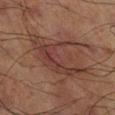Impression:
The lesion was tiled from a total-body skin photograph and was not biopsied.
Acquisition and patient details:
The tile uses cross-polarized illumination. A 15 mm close-up extracted from a 3D total-body photography capture. The patient is a male aged around 70. The lesion-visualizer software estimated an average lesion color of about L≈30 a*≈17 b*≈20 (CIELAB), roughly 6 lightness units darker than nearby skin, and a normalized lesion–skin contrast near 6.5. And it measured a nevus-likeness score of about 5/100 and lesion-presence confidence of about 65/100. On the right lower leg.